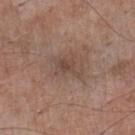This lesion was catalogued during total-body skin photography and was not selected for biopsy. A 15 mm close-up tile from a total-body photography series done for melanoma screening. Measured at roughly 3.5 mm in maximum diameter. A male subject, in their mid- to late 50s. On the left lower leg.